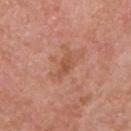follow-up=total-body-photography surveillance lesion; no biopsy | image=total-body-photography crop, ~15 mm field of view | subject=male, aged 68–72 | anatomic site=the chest.